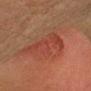Clinical impression: Captured during whole-body skin photography for melanoma surveillance; the lesion was not biopsied. Clinical summary: Imaged with cross-polarized lighting. A 15 mm crop from a total-body photograph taken for skin-cancer surveillance. Located on the head or neck. The patient is a female aged 38 to 42.